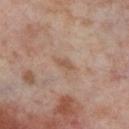Notes:
- follow-up · total-body-photography surveillance lesion; no biopsy
- size · about 2.5 mm
- imaging modality · total-body-photography crop, ~15 mm field of view
- patient · female, roughly 55 years of age
- illumination · cross-polarized
- location · the leg
- automated metrics · a lesion area of about 3.5 mm² and two-axis asymmetry of about 0.35; lesion-presence confidence of about 100/100